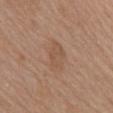<case>
  <biopsy_status>not biopsied; imaged during a skin examination</biopsy_status>
  <patient>
    <sex>female</sex>
    <age_approx>70</age_approx>
  </patient>
  <site>mid back</site>
  <image>
    <source>total-body photography crop</source>
    <field_of_view_mm>15</field_of_view_mm>
  </image>
  <lesion_size>
    <long_diameter_mm_approx>4.5</long_diameter_mm_approx>
  </lesion_size>
</case>